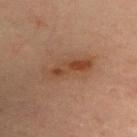notes: imaged on a skin check; not biopsied | acquisition: ~15 mm tile from a whole-body skin photo | lesion diameter: about 5.5 mm | illumination: cross-polarized illumination | body site: the chest | patient: female, about 30 years old.